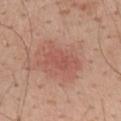workup=total-body-photography surveillance lesion; no biopsy
subject=male, aged 38–42
tile lighting=white-light
location=the mid back
image source=~15 mm crop, total-body skin-cancer survey